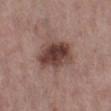Notes:
* location: the left lower leg
* patient: female, in their mid- to late 60s
* TBP lesion metrics: an eccentricity of roughly 0.7 and two-axis asymmetry of about 0.2; a lesion color around L≈42 a*≈19 b*≈22 in CIELAB and roughly 14 lightness units darker than nearby skin; a border-irregularity rating of about 2/10, internal color variation of about 7 on a 0–10 scale, and a peripheral color-asymmetry measure near 2.5
* acquisition: total-body-photography crop, ~15 mm field of view
* lighting: white-light
* lesion diameter: about 5.5 mm